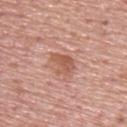| field | value |
|---|---|
| notes | catalogued during a skin exam; not biopsied |
| body site | the upper back |
| automated metrics | an average lesion color of about L≈56 a*≈24 b*≈30 (CIELAB), about 9 CIELAB-L* units darker than the surrounding skin, and a lesion-to-skin contrast of about 7 (normalized; higher = more distinct); a nevus-likeness score of about 10/100 and a lesion-detection confidence of about 100/100 |
| patient | male, about 75 years old |
| tile lighting | white-light |
| imaging modality | ~15 mm tile from a whole-body skin photo |